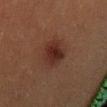Imaged during a routine full-body skin examination; the lesion was not biopsied and no histopathology is available. A roughly 15 mm field-of-view crop from a total-body skin photograph. Automated image analysis of the tile measured a lesion-to-skin contrast of about 9 (normalized; higher = more distinct). The analysis additionally found a border-irregularity rating of about 2/10, internal color variation of about 3.5 on a 0–10 scale, and peripheral color asymmetry of about 1. And it measured an automated nevus-likeness rating near 85 out of 100 and lesion-presence confidence of about 100/100. The tile uses cross-polarized illumination. A male patient, approximately 40 years of age. From the mid back. The recorded lesion diameter is about 4 mm.